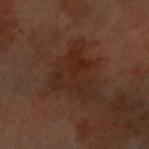Assessment:
The lesion was tiled from a total-body skin photograph and was not biopsied.
Background:
Automated image analysis of the tile measured a border-irregularity rating of about 6.5/10, a color-variation rating of about 4.5/10, and radial color variation of about 1.5. A female subject, approximately 60 years of age. A 15 mm close-up tile from a total-body photography series done for melanoma screening. Imaged with cross-polarized lighting. Longest diameter approximately 6 mm. Located on the front of the torso.